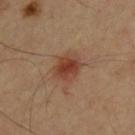Q: Is there a histopathology result?
A: no biopsy performed (imaged during a skin exam)
Q: Illumination type?
A: cross-polarized illumination
Q: Who is the patient?
A: male, aged 63 to 67
Q: Automated lesion metrics?
A: a footprint of about 8 mm², a shape eccentricity near 0.55, and a symmetry-axis asymmetry near 0.2; a lesion color around L≈41 a*≈23 b*≈30 in CIELAB and about 10 CIELAB-L* units darker than the surrounding skin; a border-irregularity rating of about 2/10, a color-variation rating of about 4/10, and peripheral color asymmetry of about 1
Q: Lesion size?
A: about 3.5 mm
Q: What kind of image is this?
A: 15 mm crop, total-body photography
Q: Where on the body is the lesion?
A: the upper back